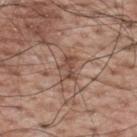{"lesion_size": {"long_diameter_mm_approx": 3.0}, "site": "upper back", "lighting": "white-light", "automated_metrics": {"area_mm2_approx": 4.5, "shape_asymmetry": 0.45, "nevus_likeness_0_100": 0, "lesion_detection_confidence_0_100": 95}, "patient": {"sex": "male", "age_approx": 70}, "image": {"source": "total-body photography crop", "field_of_view_mm": 15}}